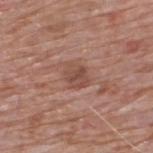Q: Was this lesion biopsied?
A: imaged on a skin check; not biopsied
Q: Where on the body is the lesion?
A: the upper back
Q: Automated lesion metrics?
A: an area of roughly 5 mm², an outline eccentricity of about 0.5 (0 = round, 1 = elongated), and a shape-asymmetry score of about 0.15 (0 = symmetric); a border-irregularity index near 1.5/10 and a peripheral color-asymmetry measure near 1; a nevus-likeness score of about 10/100 and lesion-presence confidence of about 100/100
Q: Lesion size?
A: about 2.5 mm
Q: How was the tile lit?
A: white-light
Q: How was this image acquired?
A: 15 mm crop, total-body photography
Q: Patient demographics?
A: male, about 60 years old